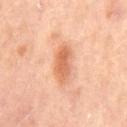Clinical impression: Captured during whole-body skin photography for melanoma surveillance; the lesion was not biopsied. Image and clinical context: A 15 mm close-up tile from a total-body photography series done for melanoma screening. Located on the right thigh. The lesion-visualizer software estimated a lesion area of about 8 mm², an eccentricity of roughly 0.9, and a symmetry-axis asymmetry near 0.2. And it measured a color-variation rating of about 3.5/10 and radial color variation of about 1. Approximately 4.5 mm at its widest. A female patient roughly 50 years of age.